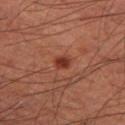workup: no biopsy performed (imaged during a skin exam) | image: 15 mm crop, total-body photography | illumination: cross-polarized | patient: male, about 60 years old | automated lesion analysis: a lesion area of about 3 mm², an outline eccentricity of about 0.55 (0 = round, 1 = elongated), and two-axis asymmetry of about 0.25; a nevus-likeness score of about 95/100 and a detector confidence of about 100 out of 100 that the crop contains a lesion | site: the left thigh | lesion size: ≈2 mm.